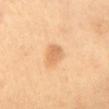The lesion was tiled from a total-body skin photograph and was not biopsied.
Automated tile analysis of the lesion measured border irregularity of about 2 on a 0–10 scale and a color-variation rating of about 1.5/10. And it measured an automated nevus-likeness rating near 55 out of 100 and a detector confidence of about 100 out of 100 that the crop contains a lesion.
The subject is a female aged around 60.
Measured at roughly 3 mm in maximum diameter.
This is a cross-polarized tile.
A lesion tile, about 15 mm wide, cut from a 3D total-body photograph.
The lesion is located on the mid back.